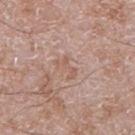biopsy_status: not biopsied; imaged during a skin examination
lesion_size:
  long_diameter_mm_approx: 3.0
patient:
  sex: male
  age_approx: 60
image:
  source: total-body photography crop
  field_of_view_mm: 15
site: right lower leg
lighting: white-light